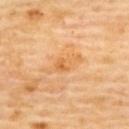Clinical impression:
Imaged during a routine full-body skin examination; the lesion was not biopsied and no histopathology is available.
Image and clinical context:
A close-up tile cropped from a whole-body skin photograph, about 15 mm across. A female patient, aged 58 to 62. Automated image analysis of the tile measured a shape eccentricity near 0.9. And it measured an average lesion color of about L≈68 a*≈24 b*≈46 (CIELAB) and roughly 8 lightness units darker than nearby skin. The lesion's longest dimension is about 4.5 mm. Located on the upper back. Captured under cross-polarized illumination.